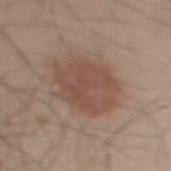The tile uses white-light illumination. A 15 mm crop from a total-body photograph taken for skin-cancer surveillance. About 7 mm across. A male subject, aged 43 to 47. The lesion-visualizer software estimated a lesion area of about 25 mm², a shape eccentricity near 0.65, and a symmetry-axis asymmetry near 0.15. And it measured an average lesion color of about L≈49 a*≈18 b*≈25 (CIELAB), roughly 9 lightness units darker than nearby skin, and a normalized lesion–skin contrast near 6.5. On the mid back.